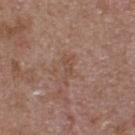Q: Was this lesion biopsied?
A: imaged on a skin check; not biopsied